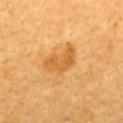Imaged during a routine full-body skin examination; the lesion was not biopsied and no histopathology is available.
About 4.5 mm across.
A female patient about 50 years old.
Located on the upper back.
This is a cross-polarized tile.
A roughly 15 mm field-of-view crop from a total-body skin photograph.